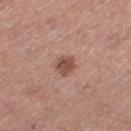Q: Is there a histopathology result?
A: total-body-photography surveillance lesion; no biopsy
Q: Lesion location?
A: the left thigh
Q: Who is the patient?
A: female, roughly 40 years of age
Q: What did automated image analysis measure?
A: a lesion area of about 4.5 mm², an outline eccentricity of about 0.7 (0 = round, 1 = elongated), and two-axis asymmetry of about 0.3; a mean CIELAB color near L≈50 a*≈22 b*≈26 and a lesion-to-skin contrast of about 8.5 (normalized; higher = more distinct); a classifier nevus-likeness of about 85/100 and a lesion-detection confidence of about 100/100
Q: What lighting was used for the tile?
A: white-light
Q: What is the imaging modality?
A: total-body-photography crop, ~15 mm field of view
Q: How large is the lesion?
A: about 3 mm